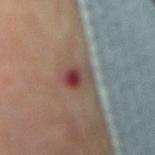| feature | finding |
|---|---|
| workup | catalogued during a skin exam; not biopsied |
| imaging modality | ~15 mm tile from a whole-body skin photo |
| subject | male, approximately 70 years of age |
| site | the lower back |